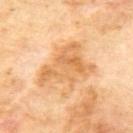Assessment: This lesion was catalogued during total-body skin photography and was not selected for biopsy. Background: From the mid back. A male subject approximately 70 years of age. The recorded lesion diameter is about 6.5 mm. A region of skin cropped from a whole-body photographic capture, roughly 15 mm wide. Imaged with cross-polarized lighting.